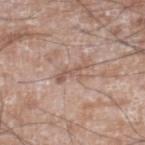Clinical impression: No biopsy was performed on this lesion — it was imaged during a full skin examination and was not determined to be concerning. Acquisition and patient details: The tile uses white-light illumination. From the leg. About 3.5 mm across. A male subject, aged around 60. Cropped from a total-body skin-imaging series; the visible field is about 15 mm. An algorithmic analysis of the crop reported a border-irregularity rating of about 7/10 and a within-lesion color-variation index near 0/10.